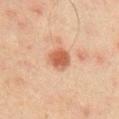follow-up: no biopsy performed (imaged during a skin exam) | lesion diameter: ~3 mm (longest diameter) | site: the chest | subject: male, aged approximately 45 | image source: ~15 mm tile from a whole-body skin photo | illumination: cross-polarized.